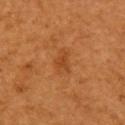{"biopsy_status": "not biopsied; imaged during a skin examination", "lesion_size": {"long_diameter_mm_approx": 3.0}, "site": "right upper arm", "patient": {"sex": "female", "age_approx": 55}, "lighting": "cross-polarized", "image": {"source": "total-body photography crop", "field_of_view_mm": 15}}